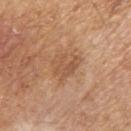biopsy status: imaged on a skin check; not biopsied
site: the right upper arm
subject: male, approximately 65 years of age
imaging modality: ~15 mm tile from a whole-body skin photo